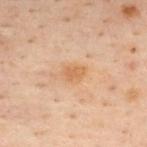  biopsy_status: not biopsied; imaged during a skin examination
  automated_metrics:
    cielab_L: 52
    cielab_a: 18
    cielab_b: 33
    vs_skin_contrast_norm: 6.0
  lesion_size:
    long_diameter_mm_approx: 2.5
  lighting: cross-polarized
  image:
    source: total-body photography crop
    field_of_view_mm: 15
  site: mid back
  patient:
    sex: male
    age_approx: 50